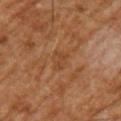Findings:
* follow-up: no biopsy performed (imaged during a skin exam)
* lighting: cross-polarized illumination
* subject: male, aged 63–67
* site: the left upper arm
* size: about 3 mm
* acquisition: ~15 mm crop, total-body skin-cancer survey